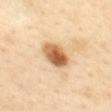Q: Is there a histopathology result?
A: catalogued during a skin exam; not biopsied
Q: What is the lesion's diameter?
A: ≈4.5 mm
Q: What is the anatomic site?
A: the mid back
Q: Patient demographics?
A: female, approximately 40 years of age
Q: Illumination type?
A: cross-polarized
Q: What is the imaging modality?
A: total-body-photography crop, ~15 mm field of view